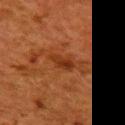notes: catalogued during a skin exam; not biopsied
imaging modality: ~15 mm tile from a whole-body skin photo
anatomic site: the upper back
subject: female, approximately 50 years of age